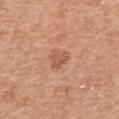Case summary:
– image — ~15 mm tile from a whole-body skin photo
– site — the upper back
– size — about 2.5 mm
– automated lesion analysis — a mean CIELAB color near L≈56 a*≈23 b*≈33, a lesion–skin lightness drop of about 9, and a normalized lesion–skin contrast near 6; a nevus-likeness score of about 5/100 and a lesion-detection confidence of about 100/100
– tile lighting — white-light illumination
– patient — female, aged around 60